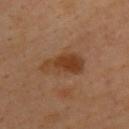Q: Was this lesion biopsied?
A: no biopsy performed (imaged during a skin exam)
Q: Who is the patient?
A: male, approximately 40 years of age
Q: What is the lesion's diameter?
A: about 5 mm
Q: What kind of image is this?
A: total-body-photography crop, ~15 mm field of view
Q: What is the anatomic site?
A: the back
Q: What lighting was used for the tile?
A: cross-polarized